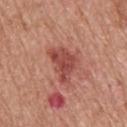This lesion was catalogued during total-body skin photography and was not selected for biopsy. Imaged with white-light lighting. The subject is a male aged approximately 55. The lesion is on the upper back. A 15 mm crop from a total-body photograph taken for skin-cancer surveillance. An algorithmic analysis of the crop reported an area of roughly 10 mm². Longest diameter approximately 4.5 mm.